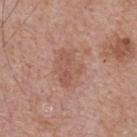Part of a total-body skin-imaging series; this lesion was reviewed on a skin check and was not flagged for biopsy. The lesion is on the upper back. The patient is a male aged 63 to 67. A roughly 15 mm field-of-view crop from a total-body skin photograph.